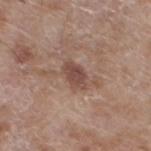The tile uses white-light illumination.
From the left lower leg.
A close-up tile cropped from a whole-body skin photograph, about 15 mm across.
A male patient, roughly 60 years of age.